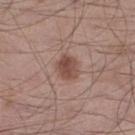Imaged during a routine full-body skin examination; the lesion was not biopsied and no histopathology is available.
On the right lower leg.
The subject is a male aged approximately 55.
A lesion tile, about 15 mm wide, cut from a 3D total-body photograph.